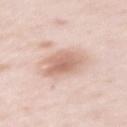Q: Was this lesion biopsied?
A: total-body-photography surveillance lesion; no biopsy